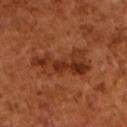biopsy status: catalogued during a skin exam; not biopsied | automated lesion analysis: an area of roughly 16 mm², a shape eccentricity near 0.9, and a shape-asymmetry score of about 0.4 (0 = symmetric); an automated nevus-likeness rating near 30 out of 100 and a detector confidence of about 100 out of 100 that the crop contains a lesion | image source: ~15 mm crop, total-body skin-cancer survey | patient: male, aged approximately 65 | illumination: cross-polarized illumination.